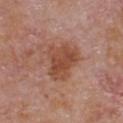notes = imaged on a skin check; not biopsied.